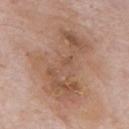The lesion was photographed on a routine skin check and not biopsied; there is no pathology result.
A lesion tile, about 15 mm wide, cut from a 3D total-body photograph.
A male subject aged around 75.
Measured at roughly 11 mm in maximum diameter.
Located on the mid back.
Automated tile analysis of the lesion measured an area of roughly 49 mm², an eccentricity of roughly 0.8, and a shape-asymmetry score of about 0.4 (0 = symmetric). And it measured border irregularity of about 6 on a 0–10 scale and peripheral color asymmetry of about 2. It also reported an automated nevus-likeness rating near 15 out of 100 and a detector confidence of about 100 out of 100 that the crop contains a lesion.